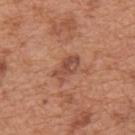A male patient, aged around 65. This is a white-light tile. Measured at roughly 3.5 mm in maximum diameter. From the back. A 15 mm close-up tile from a total-body photography series done for melanoma screening.